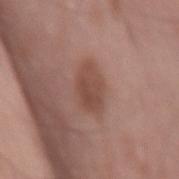Captured during whole-body skin photography for melanoma surveillance; the lesion was not biopsied.
This image is a 15 mm lesion crop taken from a total-body photograph.
Located on the lower back.
Approximately 4.5 mm at its widest.
A male patient, aged approximately 50.
The lesion-visualizer software estimated an outline eccentricity of about 0.85 (0 = round, 1 = elongated) and a shape-asymmetry score of about 0.15 (0 = symmetric). The software also gave a border-irregularity rating of about 2/10, a within-lesion color-variation index near 3/10, and peripheral color asymmetry of about 1. It also reported an automated nevus-likeness rating near 65 out of 100 and a detector confidence of about 100 out of 100 that the crop contains a lesion.